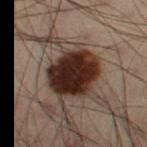Part of a total-body skin-imaging series; this lesion was reviewed on a skin check and was not flagged for biopsy. Automated image analysis of the tile measured an eccentricity of roughly 0.7 and two-axis asymmetry of about 0.15. The analysis additionally found a mean CIELAB color near L≈20 a*≈15 b*≈18 and a lesion-to-skin contrast of about 18 (normalized; higher = more distinct). A roughly 15 mm field-of-view crop from a total-body skin photograph. The tile uses cross-polarized illumination. The lesion's longest dimension is about 6 mm. The patient is a male about 55 years old. The lesion is located on the right thigh.